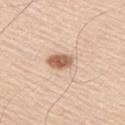Impression:
Part of a total-body skin-imaging series; this lesion was reviewed on a skin check and was not flagged for biopsy.
Acquisition and patient details:
From the upper back. Approximately 4 mm at its widest. A 15 mm close-up extracted from a 3D total-body photography capture. The patient is a male in their mid-40s. This is a white-light tile.